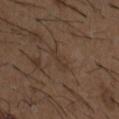Impression:
Imaged during a routine full-body skin examination; the lesion was not biopsied and no histopathology is available.
Background:
The patient is a male aged approximately 50. An algorithmic analysis of the crop reported an area of roughly 2.5 mm² and a shape eccentricity near 0.95. The software also gave a border-irregularity rating of about 5/10. It also reported a classifier nevus-likeness of about 0/100 and a lesion-detection confidence of about 65/100. The tile uses white-light illumination. The lesion is on the chest. A close-up tile cropped from a whole-body skin photograph, about 15 mm across. Longest diameter approximately 3 mm.